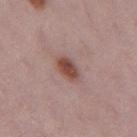This is a white-light tile. About 3 mm across. The lesion is on the left thigh. The subject is a female aged around 30. A lesion tile, about 15 mm wide, cut from a 3D total-body photograph.